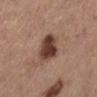biopsy_status: not biopsied; imaged during a skin examination
lesion_size:
  long_diameter_mm_approx: 3.5
patient:
  sex: female
  age_approx: 65
automated_metrics:
  cielab_L: 40
  cielab_a: 21
  cielab_b: 25
  vs_skin_darker_L: 16.0
  vs_skin_contrast_norm: 12.0
  nevus_likeness_0_100: 75
  lesion_detection_confidence_0_100: 100
site: leg
image:
  source: total-body photography crop
  field_of_view_mm: 15
lighting: white-light